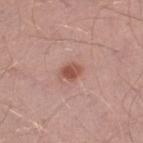Assessment: Captured during whole-body skin photography for melanoma surveillance; the lesion was not biopsied. Context: A male patient, aged 43–47. A 15 mm close-up tile from a total-body photography series done for melanoma screening. From the left thigh.